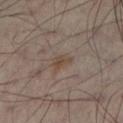<record>
  <patient>
    <sex>male</sex>
    <age_approx>50</age_approx>
  </patient>
  <lighting>cross-polarized</lighting>
  <site>left leg</site>
  <image>
    <source>total-body photography crop</source>
    <field_of_view_mm>15</field_of_view_mm>
  </image>
</record>